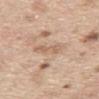{
  "biopsy_status": "not biopsied; imaged during a skin examination",
  "image": {
    "source": "total-body photography crop",
    "field_of_view_mm": 15
  },
  "lighting": "white-light",
  "site": "abdomen",
  "patient": {
    "sex": "male",
    "age_approx": 70
  },
  "lesion_size": {
    "long_diameter_mm_approx": 4.0
  }
}